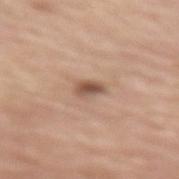Part of a total-body skin-imaging series; this lesion was reviewed on a skin check and was not flagged for biopsy. From the lower back. A 15 mm crop from a total-body photograph taken for skin-cancer surveillance. A male patient, approximately 70 years of age. The tile uses white-light illumination. About 2.5 mm across.